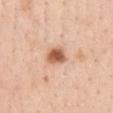Findings:
– workup: imaged on a skin check; not biopsied
– acquisition: 15 mm crop, total-body photography
– lighting: white-light illumination
– location: the chest
– patient: female, in their mid- to late 50s
– lesion diameter: about 2.5 mm
– automated lesion analysis: a symmetry-axis asymmetry near 0.2; an average lesion color of about L≈59 a*≈23 b*≈34 (CIELAB) and a lesion-to-skin contrast of about 10 (normalized; higher = more distinct); a color-variation rating of about 3.5/10; a classifier nevus-likeness of about 100/100 and lesion-presence confidence of about 100/100